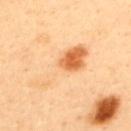Imaged during a routine full-body skin examination; the lesion was not biopsied and no histopathology is available.
The subject is a male approximately 40 years of age.
On the upper back.
Cropped from a whole-body photographic skin survey; the tile spans about 15 mm.